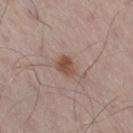Recorded during total-body skin imaging; not selected for excision or biopsy. A 15 mm crop from a total-body photograph taken for skin-cancer surveillance. Imaged with white-light lighting. The lesion is on the right thigh. Approximately 2.5 mm at its widest. A male subject aged 53 to 57.